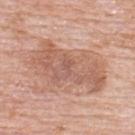No biopsy was performed on this lesion — it was imaged during a full skin examination and was not determined to be concerning. Longest diameter approximately 8.5 mm. The lesion is on the upper back. The tile uses white-light illumination. This image is a 15 mm lesion crop taken from a total-body photograph. A male patient, in their 80s. Automated image analysis of the tile measured a border-irregularity rating of about 5/10 and a peripheral color-asymmetry measure near 1.5.